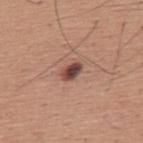Clinical summary:
Located on the mid back. Captured under white-light illumination. Measured at roughly 3 mm in maximum diameter. The patient is a male roughly 45 years of age. A 15 mm close-up extracted from a 3D total-body photography capture.